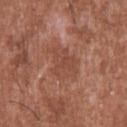Imaged during a routine full-body skin examination; the lesion was not biopsied and no histopathology is available.
Longest diameter approximately 5 mm.
A lesion tile, about 15 mm wide, cut from a 3D total-body photograph.
The patient is a male aged 43–47.
The tile uses white-light illumination.
On the upper back.
Automated image analysis of the tile measured a border-irregularity rating of about 4.5/10, a color-variation rating of about 2/10, and a peripheral color-asymmetry measure near 0.5. It also reported a nevus-likeness score of about 0/100 and a detector confidence of about 100 out of 100 that the crop contains a lesion.